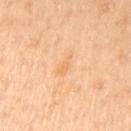This lesion was catalogued during total-body skin photography and was not selected for biopsy. The lesion is on the arm. Imaged with cross-polarized lighting. The lesion's longest dimension is about 3 mm. The lesion-visualizer software estimated a footprint of about 2.5 mm², an eccentricity of roughly 0.95, and a shape-asymmetry score of about 0.4 (0 = symmetric). Cropped from a total-body skin-imaging series; the visible field is about 15 mm. The patient is a female aged approximately 50.